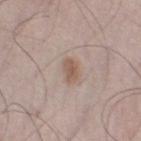Captured during whole-body skin photography for melanoma surveillance; the lesion was not biopsied. From the left lower leg. A male patient, about 50 years old. Measured at roughly 3 mm in maximum diameter. Cropped from a whole-body photographic skin survey; the tile spans about 15 mm.